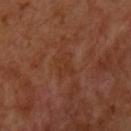notes=imaged on a skin check; not biopsied | image=~15 mm tile from a whole-body skin photo | illumination=cross-polarized | subject=male, roughly 50 years of age | lesion size=~3 mm (longest diameter) | anatomic site=the front of the torso.